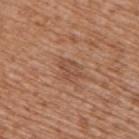Q: Was a biopsy performed?
A: total-body-photography surveillance lesion; no biopsy
Q: Automated lesion metrics?
A: a lesion area of about 4 mm²; internal color variation of about 2 on a 0–10 scale and radial color variation of about 0.5; a classifier nevus-likeness of about 0/100 and lesion-presence confidence of about 95/100
Q: What kind of image is this?
A: 15 mm crop, total-body photography
Q: Who is the patient?
A: male, aged 73–77
Q: What is the anatomic site?
A: the upper back